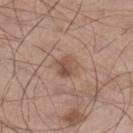notes — no biopsy performed (imaged during a skin exam) | automated lesion analysis — a mean CIELAB color near L≈51 a*≈19 b*≈27, about 9 CIELAB-L* units darker than the surrounding skin, and a lesion-to-skin contrast of about 6.5 (normalized; higher = more distinct); a color-variation rating of about 5/10 and peripheral color asymmetry of about 2; a nevus-likeness score of about 80/100 and a lesion-detection confidence of about 100/100 | subject — male, roughly 55 years of age | tile lighting — white-light illumination | imaging modality — total-body-photography crop, ~15 mm field of view | anatomic site — the right lower leg | size — about 3 mm.